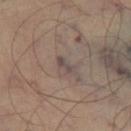Clinical impression:
No biopsy was performed on this lesion — it was imaged during a full skin examination and was not determined to be concerning.
Image and clinical context:
This is a cross-polarized tile. This image is a 15 mm lesion crop taken from a total-body photograph. A male subject about 65 years old. About 2.5 mm across. The lesion is on the right lower leg.